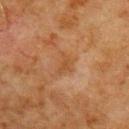Q: Was a biopsy performed?
A: imaged on a skin check; not biopsied
Q: Where on the body is the lesion?
A: the left upper arm
Q: What are the patient's age and sex?
A: male, approximately 80 years of age
Q: What kind of image is this?
A: ~15 mm crop, total-body skin-cancer survey
Q: Lesion size?
A: ≈3 mm
Q: What did automated image analysis measure?
A: a border-irregularity index near 4.5/10, internal color variation of about 1.5 on a 0–10 scale, and a peripheral color-asymmetry measure near 0.5; an automated nevus-likeness rating near 0 out of 100 and a detector confidence of about 100 out of 100 that the crop contains a lesion